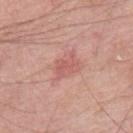Clinical impression: Imaged during a routine full-body skin examination; the lesion was not biopsied and no histopathology is available. Background: The recorded lesion diameter is about 3.5 mm. This image is a 15 mm lesion crop taken from a total-body photograph. The patient is a male approximately 60 years of age. Imaged with white-light lighting. Automated image analysis of the tile measured an average lesion color of about L≈59 a*≈27 b*≈25 (CIELAB), a lesion–skin lightness drop of about 8, and a lesion-to-skin contrast of about 5 (normalized; higher = more distinct). And it measured a border-irregularity rating of about 3/10 and peripheral color asymmetry of about 1. Located on the mid back.